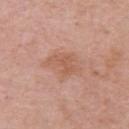Q: Is there a histopathology result?
A: total-body-photography surveillance lesion; no biopsy
Q: What kind of image is this?
A: ~15 mm tile from a whole-body skin photo
Q: What is the lesion's diameter?
A: about 4.5 mm
Q: Who is the patient?
A: female, about 45 years old
Q: Where on the body is the lesion?
A: the left upper arm
Q: What lighting was used for the tile?
A: white-light
Q: Automated lesion metrics?
A: a footprint of about 11 mm², a shape eccentricity near 0.7, and a symmetry-axis asymmetry near 0.25; border irregularity of about 3 on a 0–10 scale and radial color variation of about 1; a lesion-detection confidence of about 100/100